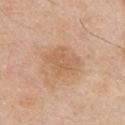biopsy_status: not biopsied; imaged during a skin examination
lighting: white-light
site: chest
lesion_size:
  long_diameter_mm_approx: 4.5
patient:
  sex: male
  age_approx: 55
automated_metrics:
  peripheral_color_asymmetry: 0.5
  nevus_likeness_0_100: 5
image:
  source: total-body photography crop
  field_of_view_mm: 15